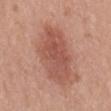diameter — about 8.5 mm; tile lighting — white-light; body site — the mid back; subject — female, roughly 40 years of age; image — 15 mm crop, total-body photography.